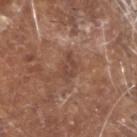Recorded during total-body skin imaging; not selected for excision or biopsy. Measured at roughly 3 mm in maximum diameter. Captured under white-light illumination. A male patient, about 80 years old. The lesion is on the head or neck. A close-up tile cropped from a whole-body skin photograph, about 15 mm across.